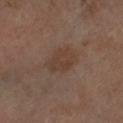follow-up: imaged on a skin check; not biopsied | automated metrics: an outline eccentricity of about 0.7 (0 = round, 1 = elongated) and two-axis asymmetry of about 0.2; a border-irregularity index near 2/10, a color-variation rating of about 2.5/10, and radial color variation of about 1; a nevus-likeness score of about 5/100 and a lesion-detection confidence of about 100/100 | anatomic site: the left lower leg | size: about 3.5 mm | subject: male, in their mid- to late 60s | image: ~15 mm crop, total-body skin-cancer survey.